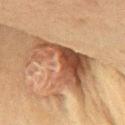Notes:
* notes — no biopsy performed (imaged during a skin exam)
* subject — male, aged around 70
* imaging modality — ~15 mm tile from a whole-body skin photo
* anatomic site — the upper back
* diameter — about 8.5 mm
* tile lighting — cross-polarized